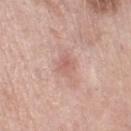Q: Was this lesion biopsied?
A: no biopsy performed (imaged during a skin exam)
Q: Illumination type?
A: white-light
Q: How was this image acquired?
A: ~15 mm crop, total-body skin-cancer survey
Q: Patient demographics?
A: female, about 55 years old
Q: What did automated image analysis measure?
A: a footprint of about 4.5 mm², an outline eccentricity of about 0.65 (0 = round, 1 = elongated), and a symmetry-axis asymmetry near 0.25; an average lesion color of about L≈61 a*≈23 b*≈25 (CIELAB), about 8 CIELAB-L* units darker than the surrounding skin, and a normalized lesion–skin contrast near 5; a border-irregularity rating of about 2.5/10
Q: Lesion location?
A: the right lower leg
Q: Lesion size?
A: ~2.5 mm (longest diameter)